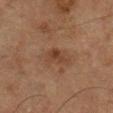Assessment:
Imaged during a routine full-body skin examination; the lesion was not biopsied and no histopathology is available.
Clinical summary:
The subject is a male in their mid- to late 80s or older. Cropped from a whole-body photographic skin survey; the tile spans about 15 mm. The lesion's longest dimension is about 2.5 mm. Located on the right lower leg.